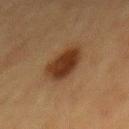workup: total-body-photography surveillance lesion; no biopsy
image-analysis metrics: a footprint of about 11 mm², a shape eccentricity near 0.75, and two-axis asymmetry of about 0.25; a color-variation rating of about 3/10 and peripheral color asymmetry of about 1
lighting: cross-polarized illumination
lesion diameter: ~5 mm (longest diameter)
imaging modality: total-body-photography crop, ~15 mm field of view
subject: female, aged 53–57
location: the mid back